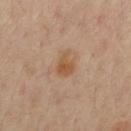{"biopsy_status": "not biopsied; imaged during a skin examination", "patient": {"sex": "male", "age_approx": 45}, "lesion_size": {"long_diameter_mm_approx": 3.0}, "image": {"source": "total-body photography crop", "field_of_view_mm": 15}, "lighting": "cross-polarized", "site": "left upper arm"}